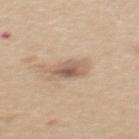biopsy status = total-body-photography surveillance lesion; no biopsy
imaging modality = ~15 mm tile from a whole-body skin photo
illumination = white-light
subject = female, roughly 40 years of age
lesion size = about 3.5 mm
location = the upper back
automated metrics = a lesion–skin lightness drop of about 11; a border-irregularity rating of about 1.5/10, internal color variation of about 5.5 on a 0–10 scale, and a peripheral color-asymmetry measure near 2